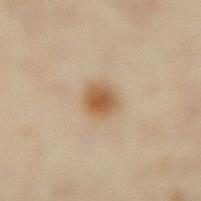workup: total-body-photography surveillance lesion; no biopsy | patient: female, about 35 years old | tile lighting: cross-polarized illumination | automated metrics: a lesion area of about 6.5 mm², an outline eccentricity of about 0.45 (0 = round, 1 = elongated), and a shape-asymmetry score of about 0.15 (0 = symmetric); a border-irregularity index near 1/10 and a color-variation rating of about 3.5/10 | diameter: about 3 mm | location: the right lower leg | acquisition: ~15 mm crop, total-body skin-cancer survey.